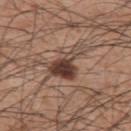Captured during whole-body skin photography for melanoma surveillance; the lesion was not biopsied. A close-up tile cropped from a whole-body skin photograph, about 15 mm across. The lesion's longest dimension is about 5 mm. The subject is a male about 60 years old. Captured under white-light illumination. From the upper back.